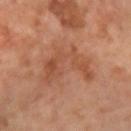Notes:
* notes — total-body-photography surveillance lesion; no biopsy
* size — ≈7.5 mm
* lighting — cross-polarized illumination
* subject — female, aged 63–67
* anatomic site — the left forearm
* image source — ~15 mm crop, total-body skin-cancer survey